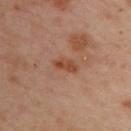{
  "biopsy_status": "not biopsied; imaged during a skin examination",
  "site": "upper back",
  "image": {
    "source": "total-body photography crop",
    "field_of_view_mm": 15
  },
  "automated_metrics": {
    "area_mm2_approx": 4.0,
    "cielab_L": 47,
    "cielab_a": 25,
    "cielab_b": 32,
    "vs_skin_darker_L": 9.0,
    "vs_skin_contrast_norm": 7.5,
    "color_variation_0_10": 4.5,
    "peripheral_color_asymmetry": 1.5,
    "lesion_detection_confidence_0_100": 100
  },
  "lesion_size": {
    "long_diameter_mm_approx": 3.0
  },
  "patient": {
    "sex": "female",
    "age_approx": 40
  },
  "lighting": "cross-polarized"
}